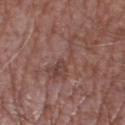The lesion was photographed on a routine skin check and not biopsied; there is no pathology result.
The lesion's longest dimension is about 7 mm.
From the right thigh.
A male patient, in their mid- to late 70s.
A close-up tile cropped from a whole-body skin photograph, about 15 mm across.